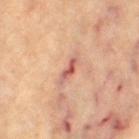Impression: This lesion was catalogued during total-body skin photography and was not selected for biopsy. Image and clinical context: A region of skin cropped from a whole-body photographic capture, roughly 15 mm wide. Imaged with cross-polarized lighting. The subject is a female aged around 65. About 4 mm across. Located on the left thigh.